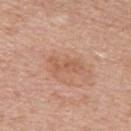biopsy status — total-body-photography surveillance lesion; no biopsy
size — ~4 mm (longest diameter)
imaging modality — ~15 mm crop, total-body skin-cancer survey
body site — the upper back
image-analysis metrics — a lesion area of about 4.5 mm², a shape eccentricity near 0.9, and two-axis asymmetry of about 0.45; an average lesion color of about L≈57 a*≈22 b*≈32 (CIELAB) and roughly 7 lightness units darker than nearby skin; a within-lesion color-variation index near 0.5/10 and radial color variation of about 0; a classifier nevus-likeness of about 0/100 and lesion-presence confidence of about 100/100
patient — male, in their mid- to late 60s